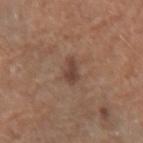| field | value |
|---|---|
| follow-up | imaged on a skin check; not biopsied |
| patient | female, aged around 60 |
| image source | 15 mm crop, total-body photography |
| site | the arm |